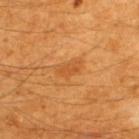Q: Is there a histopathology result?
A: total-body-photography surveillance lesion; no biopsy
Q: Illumination type?
A: cross-polarized illumination
Q: Patient demographics?
A: male, roughly 60 years of age
Q: What is the anatomic site?
A: the upper back
Q: What is the imaging modality?
A: total-body-photography crop, ~15 mm field of view
Q: Automated lesion metrics?
A: a footprint of about 4 mm² and an outline eccentricity of about 0.85 (0 = round, 1 = elongated); border irregularity of about 3 on a 0–10 scale, internal color variation of about 1.5 on a 0–10 scale, and peripheral color asymmetry of about 0.5
Q: How large is the lesion?
A: about 3 mm